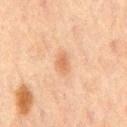Notes:
- notes · catalogued during a skin exam; not biopsied
- location · the mid back
- illumination · cross-polarized
- subject · male, aged around 65
- image · ~15 mm tile from a whole-body skin photo
- diameter · about 2.5 mm